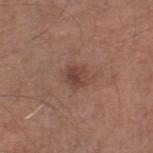{
  "biopsy_status": "not biopsied; imaged during a skin examination",
  "lesion_size": {
    "long_diameter_mm_approx": 2.5
  },
  "patient": {
    "sex": "male",
    "age_approx": 65
  },
  "lighting": "white-light",
  "image": {
    "source": "total-body photography crop",
    "field_of_view_mm": 15
  },
  "automated_metrics": {
    "area_mm2_approx": 5.0,
    "shape_asymmetry": 0.2,
    "border_irregularity_0_10": 2.0,
    "color_variation_0_10": 4.0,
    "peripheral_color_asymmetry": 1.5,
    "nevus_likeness_0_100": 60,
    "lesion_detection_confidence_0_100": 100
  },
  "site": "right thigh"
}